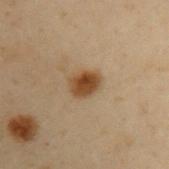{
  "biopsy_status": "not biopsied; imaged during a skin examination",
  "lighting": "cross-polarized",
  "lesion_size": {
    "long_diameter_mm_approx": 3.0
  },
  "site": "arm",
  "patient": {
    "sex": "male",
    "age_approx": 55
  },
  "image": {
    "source": "total-body photography crop",
    "field_of_view_mm": 15
  }
}